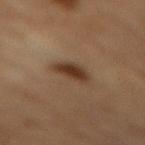Q: Was this lesion biopsied?
A: imaged on a skin check; not biopsied
Q: What are the patient's age and sex?
A: male, roughly 85 years of age or older
Q: What kind of image is this?
A: ~15 mm tile from a whole-body skin photo
Q: What lighting was used for the tile?
A: cross-polarized
Q: What is the lesion's diameter?
A: ~4 mm (longest diameter)
Q: What is the anatomic site?
A: the mid back